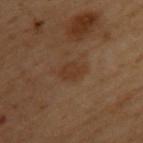Recorded during total-body skin imaging; not selected for excision or biopsy. About 3 mm across. The patient is a female about 60 years old. The lesion is located on the back. This image is a 15 mm lesion crop taken from a total-body photograph. An algorithmic analysis of the crop reported border irregularity of about 2.5 on a 0–10 scale, a color-variation rating of about 1/10, and radial color variation of about 0.5. And it measured an automated nevus-likeness rating near 5 out of 100 and a detector confidence of about 100 out of 100 that the crop contains a lesion.